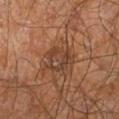{
  "site": "right leg",
  "patient": {
    "sex": "male",
    "age_approx": 60
  },
  "image": {
    "source": "total-body photography crop",
    "field_of_view_mm": 15
  },
  "lesion_size": {
    "long_diameter_mm_approx": 3.5
  }
}